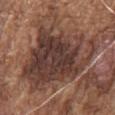| key | value |
|---|---|
| follow-up | catalogued during a skin exam; not biopsied |